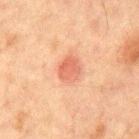Q: Was a biopsy performed?
A: no biopsy performed (imaged during a skin exam)
Q: What are the patient's age and sex?
A: male, approximately 65 years of age
Q: How was this image acquired?
A: ~15 mm crop, total-body skin-cancer survey
Q: Where on the body is the lesion?
A: the chest
Q: Lesion size?
A: about 3 mm
Q: What lighting was used for the tile?
A: cross-polarized